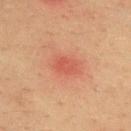A male patient aged around 35. About 3 mm across. On the upper back. Captured under cross-polarized illumination. Automated tile analysis of the lesion measured an automated nevus-likeness rating near 0 out of 100 and a lesion-detection confidence of about 100/100. A region of skin cropped from a whole-body photographic capture, roughly 15 mm wide.